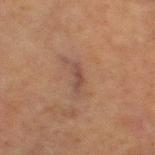* imaging modality · total-body-photography crop, ~15 mm field of view
* patient · male, about 70 years old
* body site · the right thigh